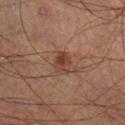The lesion was tiled from a total-body skin photograph and was not biopsied. A male subject, aged approximately 50. Longest diameter approximately 2.5 mm. Imaged with cross-polarized lighting. The lesion is located on the right lower leg. A close-up tile cropped from a whole-body skin photograph, about 15 mm across.